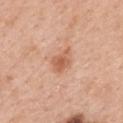workup: imaged on a skin check; not biopsied
body site: the mid back
image-analysis metrics: a footprint of about 5.5 mm² and an outline eccentricity of about 0.65 (0 = round, 1 = elongated); a mean CIELAB color near L≈60 a*≈24 b*≈34 and a lesion-to-skin contrast of about 7 (normalized; higher = more distinct); an automated nevus-likeness rating near 60 out of 100
subject: female, aged 38 to 42
image source: ~15 mm tile from a whole-body skin photo